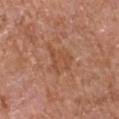Part of a total-body skin-imaging series; this lesion was reviewed on a skin check and was not flagged for biopsy. The subject is a male about 65 years old. A close-up tile cropped from a whole-body skin photograph, about 15 mm across. The lesion is on the left upper arm.